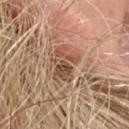The lesion was tiled from a total-body skin photograph and was not biopsied. The lesion is on the head or neck. A female subject in their mid- to late 60s. A 15 mm close-up tile from a total-body photography series done for melanoma screening. Automated tile analysis of the lesion measured a lesion area of about 9 mm², an eccentricity of roughly 0.75, and a shape-asymmetry score of about 0.35 (0 = symmetric). The analysis additionally found a classifier nevus-likeness of about 5/100. Longest diameter approximately 4.5 mm. This is a white-light tile.